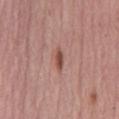The lesion was photographed on a routine skin check and not biopsied; there is no pathology result. Approximately 2.5 mm at its widest. This image is a 15 mm lesion crop taken from a total-body photograph. A female patient in their mid-60s. Located on the mid back.